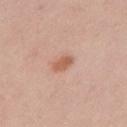  lighting: white-light
  automated_metrics:
    area_mm2_approx: 4.0
    eccentricity: 0.8
    shape_asymmetry: 0.2
  image:
    source: total-body photography crop
    field_of_view_mm: 15
  patient:
    sex: female
    age_approx: 25
  site: left upper arm